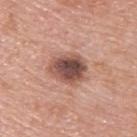Acquisition and patient details: A male patient aged approximately 80. Located on the upper back. A 15 mm close-up extracted from a 3D total-body photography capture.